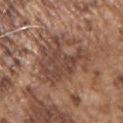  biopsy_status: not biopsied; imaged during a skin examination
  lesion_size:
    long_diameter_mm_approx: 6.5
  site: arm
  lighting: white-light
  patient:
    sex: male
    age_approx: 75
  automated_metrics:
    vs_skin_darker_L: 9.0
    vs_skin_contrast_norm: 7.0
    border_irregularity_0_10: 7.5
    peripheral_color_asymmetry: 1.5
    lesion_detection_confidence_0_100: 80
  image:
    source: total-body photography crop
    field_of_view_mm: 15